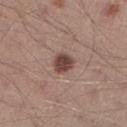No biopsy was performed on this lesion — it was imaged during a full skin examination and was not determined to be concerning. The lesion is located on the right lower leg. Cropped from a total-body skin-imaging series; the visible field is about 15 mm. The total-body-photography lesion software estimated an eccentricity of roughly 0.35 and two-axis asymmetry of about 0.15. The analysis additionally found an average lesion color of about L≈43 a*≈19 b*≈22 (CIELAB), about 14 CIELAB-L* units darker than the surrounding skin, and a lesion-to-skin contrast of about 10.5 (normalized; higher = more distinct). And it measured a border-irregularity rating of about 1.5/10 and radial color variation of about 1. And it measured an automated nevus-likeness rating near 85 out of 100 and a detector confidence of about 100 out of 100 that the crop contains a lesion. A male patient, aged around 40. Approximately 2.5 mm at its widest.